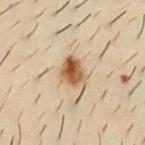| feature | finding |
|---|---|
| follow-up | catalogued during a skin exam; not biopsied |